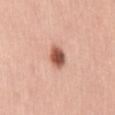Captured under white-light illumination. The patient is a male aged around 55. A 15 mm close-up extracted from a 3D total-body photography capture. The lesion is located on the back. Longest diameter approximately 3 mm.